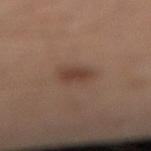  biopsy_status: not biopsied; imaged during a skin examination
  patient:
    sex: male
    age_approx: 50
  image:
    source: total-body photography crop
    field_of_view_mm: 15
  automated_metrics:
    area_mm2_approx: 5.0
    eccentricity: 0.55
    cielab_L: 39
    cielab_a: 17
    cielab_b: 24
    vs_skin_darker_L: 8.0
    vs_skin_contrast_norm: 7.0
    lesion_detection_confidence_0_100: 100
  lesion_size:
    long_diameter_mm_approx: 2.5
  site: left leg